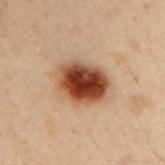Recorded during total-body skin imaging; not selected for excision or biopsy.
The patient is a male aged around 50.
The lesion's longest dimension is about 5.5 mm.
Automated image analysis of the tile measured a lesion color around L≈38 a*≈20 b*≈28 in CIELAB and about 17 CIELAB-L* units darker than the surrounding skin. The analysis additionally found border irregularity of about 1.5 on a 0–10 scale, a within-lesion color-variation index near 9/10, and a peripheral color-asymmetry measure near 2.5.
A close-up tile cropped from a whole-body skin photograph, about 15 mm across.
Located on the left arm.
The tile uses cross-polarized illumination.